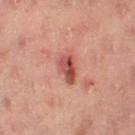Q: Is there a histopathology result?
A: catalogued during a skin exam; not biopsied
Q: Patient demographics?
A: female, aged 53 to 57
Q: What kind of image is this?
A: ~15 mm crop, total-body skin-cancer survey
Q: Lesion location?
A: the left thigh
Q: Automated lesion metrics?
A: a lesion area of about 6.5 mm², an outline eccentricity of about 0.85 (0 = round, 1 = elongated), and a symmetry-axis asymmetry near 0.3; a mean CIELAB color near L≈42 a*≈26 b*≈24, roughly 11 lightness units darker than nearby skin, and a normalized lesion–skin contrast near 9; a classifier nevus-likeness of about 5/100 and lesion-presence confidence of about 100/100